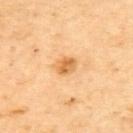Image and clinical context: The lesion is on the upper back. Imaged with cross-polarized lighting. A male subject, aged 63 to 67. Approximately 2.5 mm at its widest. A 15 mm close-up extracted from a 3D total-body photography capture. Automated image analysis of the tile measured an area of roughly 4.5 mm², an outline eccentricity of about 0.5 (0 = round, 1 = elongated), and a shape-asymmetry score of about 0.2 (0 = symmetric). And it measured an automated nevus-likeness rating near 65 out of 100 and a detector confidence of about 100 out of 100 that the crop contains a lesion.